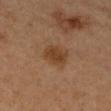This lesion was catalogued during total-body skin photography and was not selected for biopsy. A female subject, aged around 60. A roughly 15 mm field-of-view crop from a total-body skin photograph. The lesion is located on the right forearm. An algorithmic analysis of the crop reported an area of roughly 7 mm² and a shape-asymmetry score of about 0.2 (0 = symmetric). The analysis additionally found a border-irregularity rating of about 2/10, internal color variation of about 2 on a 0–10 scale, and a peripheral color-asymmetry measure near 0.5. Measured at roughly 3.5 mm in maximum diameter. The tile uses cross-polarized illumination.